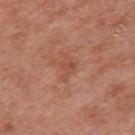Clinical impression: This lesion was catalogued during total-body skin photography and was not selected for biopsy. Image and clinical context: A roughly 15 mm field-of-view crop from a total-body skin photograph. A male patient aged 68–72. The tile uses white-light illumination. Measured at roughly 3.5 mm in maximum diameter. The lesion is on the mid back.